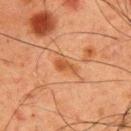The lesion was tiled from a total-body skin photograph and was not biopsied. A lesion tile, about 15 mm wide, cut from a 3D total-body photograph. Located on the upper back. A male patient, roughly 60 years of age.